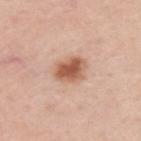* workup — no biopsy performed (imaged during a skin exam)
* tile lighting — white-light illumination
* size — ~4.5 mm (longest diameter)
* subject — male, in their 60s
* automated lesion analysis — a footprint of about 9 mm² and a symmetry-axis asymmetry near 0.15; roughly 14 lightness units darker than nearby skin; a detector confidence of about 100 out of 100 that the crop contains a lesion
* location — the upper back
* image — ~15 mm tile from a whole-body skin photo